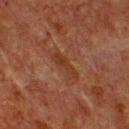Assessment:
Recorded during total-body skin imaging; not selected for excision or biopsy.
Clinical summary:
A male patient approximately 75 years of age. Approximately 4 mm at its widest. An algorithmic analysis of the crop reported an average lesion color of about L≈29 a*≈20 b*≈26 (CIELAB), roughly 6 lightness units darker than nearby skin, and a lesion-to-skin contrast of about 6.5 (normalized; higher = more distinct). And it measured a classifier nevus-likeness of about 0/100. A lesion tile, about 15 mm wide, cut from a 3D total-body photograph. From the chest.